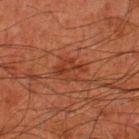Clinical summary: The lesion is on the left lower leg. The lesion-visualizer software estimated border irregularity of about 3.5 on a 0–10 scale, a within-lesion color-variation index near 3.5/10, and peripheral color asymmetry of about 1. It also reported a classifier nevus-likeness of about 5/100. Captured under cross-polarized illumination. This image is a 15 mm lesion crop taken from a total-body photograph. A male patient aged 78 to 82. The recorded lesion diameter is about 3.5 mm.